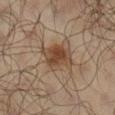Assessment: Recorded during total-body skin imaging; not selected for excision or biopsy.